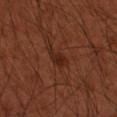- biopsy status: imaged on a skin check; not biopsied
- imaging modality: total-body-photography crop, ~15 mm field of view
- tile lighting: cross-polarized
- size: ~3 mm (longest diameter)
- patient: male, roughly 50 years of age
- site: the left forearm